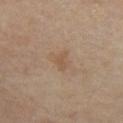Case summary:
* workup · catalogued during a skin exam; not biopsied
* tile lighting · cross-polarized illumination
* location · the front of the torso
* image-analysis metrics · an area of roughly 3 mm², an eccentricity of roughly 0.75, and two-axis asymmetry of about 0.35; a lesion–skin lightness drop of about 6 and a normalized lesion–skin contrast near 5.5; a border-irregularity index near 3.5/10, internal color variation of about 1 on a 0–10 scale, and a peripheral color-asymmetry measure near 0.5; an automated nevus-likeness rating near 0 out of 100 and lesion-presence confidence of about 100/100
* patient · female, about 50 years old
* lesion diameter · about 2.5 mm
* imaging modality · ~15 mm crop, total-body skin-cancer survey